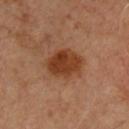Recorded during total-body skin imaging; not selected for excision or biopsy. A male subject, roughly 50 years of age. The recorded lesion diameter is about 4.5 mm. From the chest. Imaged with cross-polarized lighting. Cropped from a whole-body photographic skin survey; the tile spans about 15 mm.